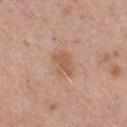Q: Was this lesion biopsied?
A: total-body-photography surveillance lesion; no biopsy
Q: What lighting was used for the tile?
A: white-light illumination
Q: What are the patient's age and sex?
A: female, about 40 years old
Q: Lesion size?
A: about 3 mm
Q: Automated lesion metrics?
A: a footprint of about 5.5 mm², an eccentricity of roughly 0.65, and a symmetry-axis asymmetry near 0.3; an average lesion color of about L≈57 a*≈21 b*≈31 (CIELAB), roughly 8 lightness units darker than nearby skin, and a normalized border contrast of about 6.5; internal color variation of about 1.5 on a 0–10 scale; an automated nevus-likeness rating near 10 out of 100 and lesion-presence confidence of about 100/100
Q: What is the imaging modality?
A: ~15 mm crop, total-body skin-cancer survey
Q: Where on the body is the lesion?
A: the right thigh